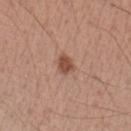biopsy status: no biopsy performed (imaged during a skin exam) | patient: male, approximately 55 years of age | acquisition: total-body-photography crop, ~15 mm field of view | tile lighting: white-light illumination | body site: the left forearm.